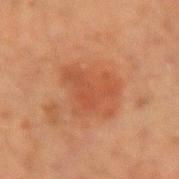Impression: The lesion was tiled from a total-body skin photograph and was not biopsied. Clinical summary: A region of skin cropped from a whole-body photographic capture, roughly 15 mm wide. Longest diameter approximately 5 mm. On the right forearm. This is a cross-polarized tile. A male subject, in their mid- to late 40s.